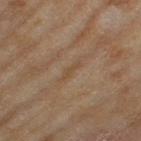Q: Was a biopsy performed?
A: no biopsy performed (imaged during a skin exam)
Q: Automated lesion metrics?
A: border irregularity of about 4 on a 0–10 scale and radial color variation of about 0
Q: Illumination type?
A: cross-polarized
Q: How was this image acquired?
A: ~15 mm tile from a whole-body skin photo
Q: Who is the patient?
A: female, aged around 60
Q: Where on the body is the lesion?
A: the left thigh